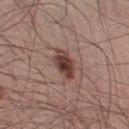biopsy status = imaged on a skin check; not biopsied | anatomic site = the right lower leg | subject = male, about 30 years old | imaging modality = ~15 mm tile from a whole-body skin photo.